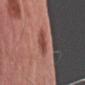* acquisition: ~15 mm crop, total-body skin-cancer survey
* body site: the arm
* subject: male, in their mid- to late 70s